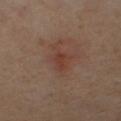This lesion was catalogued during total-body skin photography and was not selected for biopsy.
On the right leg.
The patient is a female aged 58 to 62.
A roughly 15 mm field-of-view crop from a total-body skin photograph.